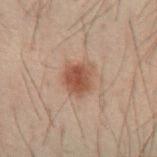Case summary:
– notes · catalogued during a skin exam; not biopsied
– patient · male, about 40 years old
– size · ≈3.5 mm
– illumination · cross-polarized illumination
– anatomic site · the left forearm
– image source · total-body-photography crop, ~15 mm field of view